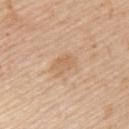TBP lesion metrics: a lesion color around L≈63 a*≈18 b*≈35 in CIELAB and a lesion–skin lightness drop of about 7; border irregularity of about 3 on a 0–10 scale, a color-variation rating of about 2/10, and peripheral color asymmetry of about 0.5 | illumination: white-light illumination | subject: male, aged 58–62 | lesion diameter: ~3 mm (longest diameter) | image: total-body-photography crop, ~15 mm field of view | body site: the back.